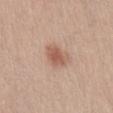Clinical impression:
This lesion was catalogued during total-body skin photography and was not selected for biopsy.
Image and clinical context:
Located on the abdomen. The patient is a female in their mid- to late 20s. A roughly 15 mm field-of-view crop from a total-body skin photograph.